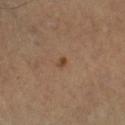Clinical impression:
The lesion was tiled from a total-body skin photograph and was not biopsied.
Context:
A male subject aged 53 to 57. A 15 mm crop from a total-body photograph taken for skin-cancer surveillance. The lesion is on the left forearm.